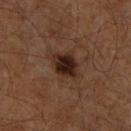Clinical impression: This lesion was catalogued during total-body skin photography and was not selected for biopsy. Background: This image is a 15 mm lesion crop taken from a total-body photograph. From the right lower leg. The subject is a male about 60 years old. Imaged with cross-polarized lighting. Measured at roughly 3.5 mm in maximum diameter. The total-body-photography lesion software estimated a color-variation rating of about 3.5/10 and a peripheral color-asymmetry measure near 1. It also reported lesion-presence confidence of about 100/100.